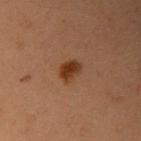This lesion was catalogued during total-body skin photography and was not selected for biopsy. A roughly 15 mm field-of-view crop from a total-body skin photograph. A female subject aged 38 to 42. The lesion is located on the arm.